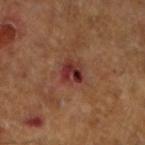The lesion was tiled from a total-body skin photograph and was not biopsied.
This is a cross-polarized tile.
Approximately 3 mm at its widest.
Located on the right forearm.
A male patient aged 58–62.
Cropped from a total-body skin-imaging series; the visible field is about 15 mm.